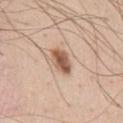biopsy_status: not biopsied; imaged during a skin examination
site: chest
patient:
  sex: male
  age_approx: 45
lighting: white-light
image:
  source: total-body photography crop
  field_of_view_mm: 15
lesion_size:
  long_diameter_mm_approx: 3.5